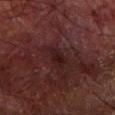Findings:
- workup: no biopsy performed (imaged during a skin exam)
- tile lighting: cross-polarized illumination
- site: the arm
- patient: male, roughly 70 years of age
- imaging modality: total-body-photography crop, ~15 mm field of view
- TBP lesion metrics: a mean CIELAB color near L≈14 a*≈18 b*≈14, roughly 5 lightness units darker than nearby skin, and a lesion-to-skin contrast of about 7.5 (normalized; higher = more distinct); a nevus-likeness score of about 0/100 and a detector confidence of about 95 out of 100 that the crop contains a lesion
- lesion size: ≈3 mm